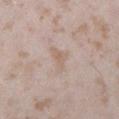{
  "biopsy_status": "not biopsied; imaged during a skin examination",
  "site": "right lower leg",
  "image": {
    "source": "total-body photography crop",
    "field_of_view_mm": 15
  },
  "lighting": "white-light",
  "patient": {
    "sex": "female",
    "age_approx": 25
  },
  "lesion_size": {
    "long_diameter_mm_approx": 3.5
  }
}